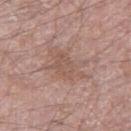Notes:
- workup — catalogued during a skin exam; not biopsied
- acquisition — 15 mm crop, total-body photography
- subject — male, approximately 60 years of age
- tile lighting — white-light illumination
- TBP lesion metrics — internal color variation of about 2 on a 0–10 scale and radial color variation of about 0.5
- diameter — ~5.5 mm (longest diameter)
- body site — the right thigh